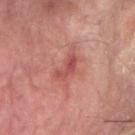No biopsy was performed on this lesion — it was imaged during a full skin examination and was not determined to be concerning. This image is a 15 mm lesion crop taken from a total-body photograph. A male patient in their mid- to late 70s. Located on the right forearm. About 3 mm across.